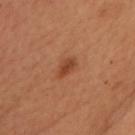workup: imaged on a skin check; not biopsied
tile lighting: cross-polarized
diameter: ≈2.5 mm
body site: the chest
patient: female, approximately 50 years of age
imaging modality: total-body-photography crop, ~15 mm field of view